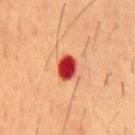Assessment:
Recorded during total-body skin imaging; not selected for excision or biopsy.
Image and clinical context:
The patient is a male about 55 years old. On the mid back. This is a cross-polarized tile. Cropped from a total-body skin-imaging series; the visible field is about 15 mm. About 3 mm across.